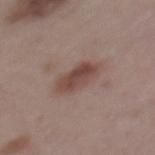body site: the mid back
tile lighting: white-light
acquisition: total-body-photography crop, ~15 mm field of view
patient: female, roughly 45 years of age
size: ≈5 mm
image-analysis metrics: a footprint of about 9 mm² and a symmetry-axis asymmetry near 0.2; a mean CIELAB color near L≈45 a*≈19 b*≈22, about 10 CIELAB-L* units darker than the surrounding skin, and a lesion-to-skin contrast of about 8 (normalized; higher = more distinct); a border-irregularity rating of about 2.5/10 and a within-lesion color-variation index near 4.5/10; a classifier nevus-likeness of about 75/100 and lesion-presence confidence of about 100/100